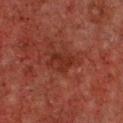The lesion was photographed on a routine skin check and not biopsied; there is no pathology result. The subject is a male aged around 50. A 15 mm crop from a total-body photograph taken for skin-cancer surveillance. The lesion is located on the chest. This is a cross-polarized tile.